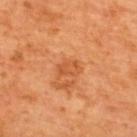A region of skin cropped from a whole-body photographic capture, roughly 15 mm wide. The subject is a male in their mid- to late 60s. From the upper back.